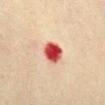{"biopsy_status": "not biopsied; imaged during a skin examination", "site": "chest", "lesion_size": {"long_diameter_mm_approx": 3.0}, "patient": {"sex": "female", "age_approx": 40}, "image": {"source": "total-body photography crop", "field_of_view_mm": 15}, "lighting": "cross-polarized"}